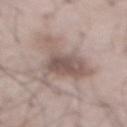| field | value |
|---|---|
| follow-up | no biopsy performed (imaged during a skin exam) |
| illumination | white-light |
| anatomic site | the abdomen |
| subject | male, about 55 years old |
| lesion size | ~8 mm (longest diameter) |
| acquisition | total-body-photography crop, ~15 mm field of view |
| automated lesion analysis | a lesion area of about 19 mm² and a symmetry-axis asymmetry near 0.5; border irregularity of about 7.5 on a 0–10 scale, a within-lesion color-variation index near 4/10, and peripheral color asymmetry of about 1; a classifier nevus-likeness of about 70/100 and a detector confidence of about 80 out of 100 that the crop contains a lesion |